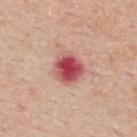The patient is a male in their mid- to late 60s. Captured under white-light illumination. A close-up tile cropped from a whole-body skin photograph, about 15 mm across. On the upper back.